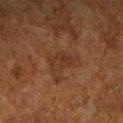workup — total-body-photography surveillance lesion; no biopsy
patient — male, roughly 60 years of age
lighting — cross-polarized illumination
lesion size — ~3.5 mm (longest diameter)
acquisition — 15 mm crop, total-body photography
body site — the arm
automated lesion analysis — a lesion color around L≈28 a*≈17 b*≈25 in CIELAB, a lesion–skin lightness drop of about 5, and a normalized border contrast of about 5.5; border irregularity of about 3 on a 0–10 scale and internal color variation of about 2.5 on a 0–10 scale